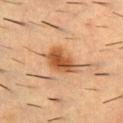Clinical impression:
This lesion was catalogued during total-body skin photography and was not selected for biopsy.
Clinical summary:
A lesion tile, about 15 mm wide, cut from a 3D total-body photograph. From the upper back. Imaged with cross-polarized lighting. The recorded lesion diameter is about 5 mm. A male subject, about 55 years old.